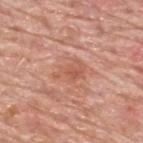| key | value |
|---|---|
| notes | no biopsy performed (imaged during a skin exam) |
| size | ≈3.5 mm |
| patient | male, about 80 years old |
| site | the upper back |
| image | 15 mm crop, total-body photography |